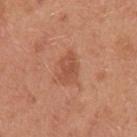Recorded during total-body skin imaging; not selected for excision or biopsy. A male patient about 30 years old. The total-body-photography lesion software estimated a lesion area of about 6 mm² and two-axis asymmetry of about 0.4. It also reported a border-irregularity index near 3.5/10, a within-lesion color-variation index near 2.5/10, and radial color variation of about 1. A 15 mm crop from a total-body photograph taken for skin-cancer surveillance. The lesion is on the left upper arm. Longest diameter approximately 3.5 mm. Captured under white-light illumination.